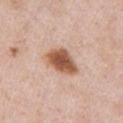<record>
  <biopsy_status>not biopsied; imaged during a skin examination</biopsy_status>
  <site>chest</site>
  <lesion_size>
    <long_diameter_mm_approx>4.5</long_diameter_mm_approx>
  </lesion_size>
  <image>
    <source>total-body photography crop</source>
    <field_of_view_mm>15</field_of_view_mm>
  </image>
  <patient>
    <sex>male</sex>
    <age_approx>50</age_approx>
  </patient>
  <automated_metrics>
    <area_mm2_approx>10.0</area_mm2_approx>
    <shape_asymmetry>0.2</shape_asymmetry>
    <vs_skin_darker_L>17.0</vs_skin_darker_L>
    <vs_skin_contrast_norm>11.0</vs_skin_contrast_norm>
  </automated_metrics>
</record>